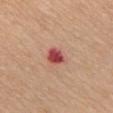Clinical impression: Imaged during a routine full-body skin examination; the lesion was not biopsied and no histopathology is available. Acquisition and patient details: A roughly 15 mm field-of-view crop from a total-body skin photograph. About 2.5 mm across. Automated tile analysis of the lesion measured a detector confidence of about 100 out of 100 that the crop contains a lesion. A female patient, in their mid-60s. Located on the chest.